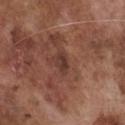biopsy_status: not biopsied; imaged during a skin examination
automated_metrics:
  area_mm2_approx: 3.0
  eccentricity: 0.8
  shape_asymmetry: 0.2
  cielab_L: 36
  cielab_a: 20
  cielab_b: 24
  vs_skin_darker_L: 8.0
  vs_skin_contrast_norm: 7.5
  nevus_likeness_0_100: 5
  lesion_detection_confidence_0_100: 95
lighting: white-light
image:
  source: total-body photography crop
  field_of_view_mm: 15
site: front of the torso
patient:
  sex: male
  age_approx: 75
lesion_size:
  long_diameter_mm_approx: 2.5